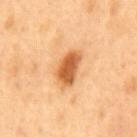Impression: Recorded during total-body skin imaging; not selected for excision or biopsy. Background: Approximately 4.5 mm at its widest. Captured under cross-polarized illumination. A lesion tile, about 15 mm wide, cut from a 3D total-body photograph. The lesion is located on the mid back. The subject is a male roughly 50 years of age.